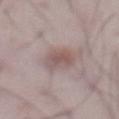Q: Is there a histopathology result?
A: imaged on a skin check; not biopsied
Q: What are the patient's age and sex?
A: male, in their 50s
Q: What kind of image is this?
A: 15 mm crop, total-body photography
Q: Lesion size?
A: ≈4 mm
Q: Illumination type?
A: white-light
Q: What is the anatomic site?
A: the abdomen